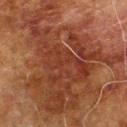Q: What kind of image is this?
A: ~15 mm tile from a whole-body skin photo
Q: Where on the body is the lesion?
A: the right upper arm
Q: What lighting was used for the tile?
A: cross-polarized
Q: Patient demographics?
A: male, about 70 years old
Q: Automated lesion metrics?
A: an average lesion color of about L≈32 a*≈22 b*≈27 (CIELAB) and a normalized border contrast of about 8
Q: Lesion size?
A: ≈12.5 mm